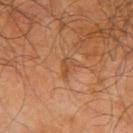{
  "biopsy_status": "not biopsied; imaged during a skin examination",
  "patient": {
    "sex": "male",
    "age_approx": 65
  },
  "site": "arm",
  "lighting": "cross-polarized",
  "lesion_size": {
    "long_diameter_mm_approx": 2.5
  },
  "image": {
    "source": "total-body photography crop",
    "field_of_view_mm": 15
  },
  "automated_metrics": {
    "cielab_L": 46,
    "cielab_a": 22,
    "cielab_b": 34,
    "vs_skin_darker_L": 7.0,
    "vs_skin_contrast_norm": 5.5,
    "nevus_likeness_0_100": 0,
    "lesion_detection_confidence_0_100": 100
  }
}